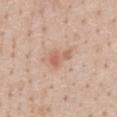This lesion was catalogued during total-body skin photography and was not selected for biopsy.
From the chest.
The tile uses white-light illumination.
A close-up tile cropped from a whole-body skin photograph, about 15 mm across.
The patient is a female roughly 45 years of age.